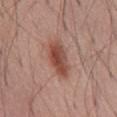biopsy status = imaged on a skin check; not biopsied | site = the abdomen | subject = male, about 55 years old | image source = total-body-photography crop, ~15 mm field of view | lighting = white-light | size = ~5 mm (longest diameter).